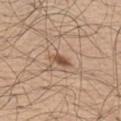biopsy_status: not biopsied; imaged during a skin examination
image:
  source: total-body photography crop
  field_of_view_mm: 15
patient:
  sex: male
  age_approx: 70
site: leg